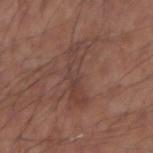follow-up=imaged on a skin check; not biopsied
image=~15 mm crop, total-body skin-cancer survey
location=the left forearm
patient=male, aged 53 to 57